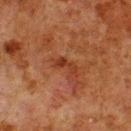Captured during whole-body skin photography for melanoma surveillance; the lesion was not biopsied.
Located on the back.
A region of skin cropped from a whole-body photographic capture, roughly 15 mm wide.
About 3 mm across.
Imaged with cross-polarized lighting.
A male subject, aged 78 to 82.